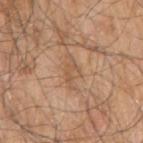notes: total-body-photography surveillance lesion; no biopsy
subject: male, aged approximately 80
image: total-body-photography crop, ~15 mm field of view
tile lighting: white-light
TBP lesion metrics: a mean CIELAB color near L≈55 a*≈19 b*≈33 and about 7 CIELAB-L* units darker than the surrounding skin; a color-variation rating of about 2/10 and radial color variation of about 0.5
lesion diameter: about 4 mm
body site: the arm